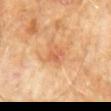Clinical impression:
Imaged during a routine full-body skin examination; the lesion was not biopsied and no histopathology is available.
Clinical summary:
The lesion's longest dimension is about 2.5 mm. A male patient, about 60 years old. Cropped from a total-body skin-imaging series; the visible field is about 15 mm. The lesion-visualizer software estimated two-axis asymmetry of about 0.35. The software also gave a border-irregularity rating of about 3.5/10, a color-variation rating of about 1/10, and peripheral color asymmetry of about 0. The lesion is on the abdomen. This is a cross-polarized tile.